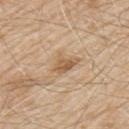Q: Illumination type?
A: white-light
Q: How large is the lesion?
A: ≈3.5 mm
Q: Where on the body is the lesion?
A: the left upper arm
Q: What kind of image is this?
A: 15 mm crop, total-body photography
Q: Who is the patient?
A: male, aged 78 to 82